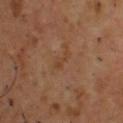* follow-up · total-body-photography surveillance lesion; no biopsy
* diameter · ≈2.5 mm
* imaging modality · ~15 mm tile from a whole-body skin photo
* automated lesion analysis · a shape eccentricity near 0.95 and a symmetry-axis asymmetry near 0.5; a lesion-detection confidence of about 100/100
* site · the upper back
* patient · male, aged around 55